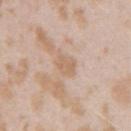Assessment:
The lesion was photographed on a routine skin check and not biopsied; there is no pathology result.
Clinical summary:
Captured under white-light illumination. A roughly 15 mm field-of-view crop from a total-body skin photograph. Approximately 2.5 mm at its widest. A female subject, aged approximately 25. On the arm. The total-body-photography lesion software estimated a footprint of about 5 mm², an outline eccentricity of about 0.5 (0 = round, 1 = elongated), and a shape-asymmetry score of about 0.25 (0 = symmetric). And it measured a color-variation rating of about 1.5/10 and a peripheral color-asymmetry measure near 0.5.